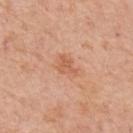{"biopsy_status": "not biopsied; imaged during a skin examination", "site": "left forearm", "image": {"source": "total-body photography crop", "field_of_view_mm": 15}, "patient": {"sex": "female", "age_approx": 65}}